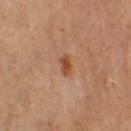Part of a total-body skin-imaging series; this lesion was reviewed on a skin check and was not flagged for biopsy. On the right thigh. Imaged with cross-polarized lighting. This image is a 15 mm lesion crop taken from a total-body photograph. Longest diameter approximately 2.5 mm. A female patient aged 48–52.